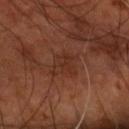Assessment:
Captured during whole-body skin photography for melanoma surveillance; the lesion was not biopsied.
Acquisition and patient details:
The lesion-visualizer software estimated an area of roughly 7 mm² and a symmetry-axis asymmetry near 0.25. It also reported a lesion color around L≈28 a*≈21 b*≈25 in CIELAB, a lesion–skin lightness drop of about 5, and a normalized border contrast of about 5. And it measured a border-irregularity rating of about 3.5/10 and radial color variation of about 0.5. Imaged with cross-polarized lighting. A 15 mm crop from a total-body photograph taken for skin-cancer surveillance. A male subject, in their mid- to late 50s. From the right forearm.